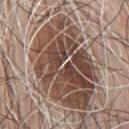notes = total-body-photography surveillance lesion; no biopsy | subject = male, about 65 years old | image = 15 mm crop, total-body photography | anatomic site = the chest.